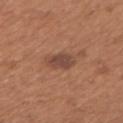  biopsy_status: not biopsied; imaged during a skin examination
  site: left upper arm
  image:
    source: total-body photography crop
    field_of_view_mm: 15
  lighting: white-light
  lesion_size:
    long_diameter_mm_approx: 3.5
  automated_metrics:
    area_mm2_approx: 6.0
    eccentricity: 0.7
    shape_asymmetry: 0.2
    cielab_L: 45
    cielab_a: 21
    cielab_b: 27
    vs_skin_darker_L: 9.0
    nevus_likeness_0_100: 55
    lesion_detection_confidence_0_100: 100
  patient:
    sex: female
    age_approx: 65